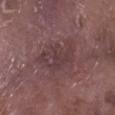Imaged during a routine full-body skin examination; the lesion was not biopsied and no histopathology is available.
A close-up tile cropped from a whole-body skin photograph, about 15 mm across.
A male subject roughly 75 years of age.
Located on the right lower leg.